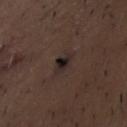Findings:
* notes: imaged on a skin check; not biopsied
* location: the chest
* automated lesion analysis: a lesion area of about 5 mm² and an eccentricity of roughly 0.65; border irregularity of about 2 on a 0–10 scale, a color-variation rating of about 5.5/10, and peripheral color asymmetry of about 1.5
* image source: 15 mm crop, total-body photography
* subject: male, in their 50s
* lesion diameter: ~3 mm (longest diameter)